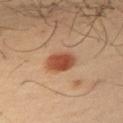workup: catalogued during a skin exam; not biopsied
location: the left upper arm
size: ~3.5 mm (longest diameter)
illumination: cross-polarized
patient: male, aged 38 to 42
image-analysis metrics: a footprint of about 7.5 mm², an eccentricity of roughly 0.6, and a shape-asymmetry score of about 0.1 (0 = symmetric)
acquisition: ~15 mm crop, total-body skin-cancer survey